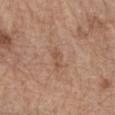Captured during whole-body skin photography for melanoma surveillance; the lesion was not biopsied. A lesion tile, about 15 mm wide, cut from a 3D total-body photograph. A male subject, aged 68–72. The lesion is on the right forearm. The total-body-photography lesion software estimated an eccentricity of roughly 0.95. And it measured an automated nevus-likeness rating near 0 out of 100.